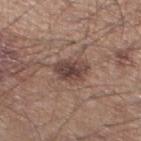Assessment: The lesion was tiled from a total-body skin photograph and was not biopsied. Context: Measured at roughly 3.5 mm in maximum diameter. A male subject aged 43 to 47. Cropped from a total-body skin-imaging series; the visible field is about 15 mm. The lesion is located on the leg.